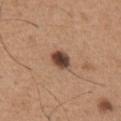Q: Is there a histopathology result?
A: no biopsy performed (imaged during a skin exam)
Q: What lighting was used for the tile?
A: white-light illumination
Q: Where on the body is the lesion?
A: the chest
Q: Patient demographics?
A: male, in their mid-60s
Q: What is the lesion's diameter?
A: ~2.5 mm (longest diameter)
Q: What kind of image is this?
A: 15 mm crop, total-body photography